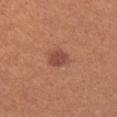– subject: female, about 25 years old
– lesion size: about 3 mm
– imaging modality: ~15 mm crop, total-body skin-cancer survey
– site: the left forearm
– illumination: white-light